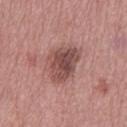The lesion was photographed on a routine skin check and not biopsied; there is no pathology result.
This image is a 15 mm lesion crop taken from a total-body photograph.
The lesion is located on the left thigh.
The subject is a female about 70 years old.